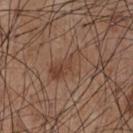No biopsy was performed on this lesion — it was imaged during a full skin examination and was not determined to be concerning.
The lesion is on the front of the torso.
A male patient approximately 55 years of age.
This image is a 15 mm lesion crop taken from a total-body photograph.
Approximately 5 mm at its widest.
Imaged with white-light lighting.